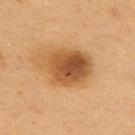notes=no biopsy performed (imaged during a skin exam) | image source=total-body-photography crop, ~15 mm field of view | body site=the upper back | patient=female, aged approximately 40.